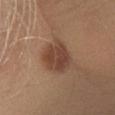– follow-up: imaged on a skin check; not biopsied
– subject: male, about 30 years old
– tile lighting: white-light illumination
– location: the right lower leg
– image: ~15 mm crop, total-body skin-cancer survey
– lesion size: ≈4 mm